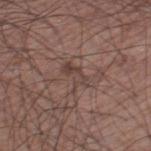• workup — no biopsy performed (imaged during a skin exam)
• tile lighting — white-light illumination
• anatomic site — the left thigh
• automated metrics — a footprint of about 5 mm² and two-axis asymmetry of about 0.5; a mean CIELAB color near L≈42 a*≈16 b*≈21 and a lesion–skin lightness drop of about 7; a classifier nevus-likeness of about 0/100 and lesion-presence confidence of about 55/100
• patient — male, approximately 60 years of age
• imaging modality — total-body-photography crop, ~15 mm field of view
• size — about 3.5 mm